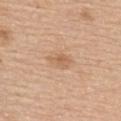{
  "biopsy_status": "not biopsied; imaged during a skin examination",
  "image": {
    "source": "total-body photography crop",
    "field_of_view_mm": 15
  },
  "site": "upper back",
  "patient": {
    "sex": "female",
    "age_approx": 65
  }
}